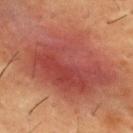Q: Was this lesion biopsied?
A: imaged on a skin check; not biopsied
Q: What kind of image is this?
A: ~15 mm tile from a whole-body skin photo
Q: How large is the lesion?
A: ~12 mm (longest diameter)
Q: Lesion location?
A: the chest
Q: Who is the patient?
A: male, in their mid- to late 50s
Q: How was the tile lit?
A: cross-polarized